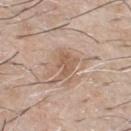Imaged during a routine full-body skin examination; the lesion was not biopsied and no histopathology is available.